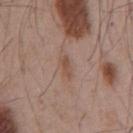* notes · no biopsy performed (imaged during a skin exam)
* image · 15 mm crop, total-body photography
* lesion size · ~2.5 mm (longest diameter)
* location · the chest
* patient · male, approximately 55 years of age